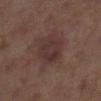The lesion was tiled from a total-body skin photograph and was not biopsied.